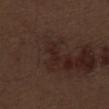Q: Where on the body is the lesion?
A: the abdomen
Q: What is the lesion's diameter?
A: ≈2.5 mm
Q: What kind of image is this?
A: 15 mm crop, total-body photography
Q: Patient demographics?
A: male, about 70 years old